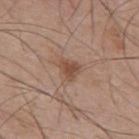Clinical impression:
This lesion was catalogued during total-body skin photography and was not selected for biopsy.
Background:
A male subject about 55 years old. The tile uses white-light illumination. From the mid back. A lesion tile, about 15 mm wide, cut from a 3D total-body photograph. The total-body-photography lesion software estimated border irregularity of about 3 on a 0–10 scale, a color-variation rating of about 2/10, and a peripheral color-asymmetry measure near 0.5. And it measured a nevus-likeness score of about 75/100 and a lesion-detection confidence of about 100/100.